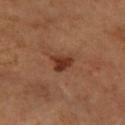Impression: No biopsy was performed on this lesion — it was imaged during a full skin examination and was not determined to be concerning. Clinical summary: A female patient aged around 65. The lesion is located on the right forearm. Automated tile analysis of the lesion measured a border-irregularity index near 4/10 and radial color variation of about 1. The software also gave an automated nevus-likeness rating near 90 out of 100 and a detector confidence of about 100 out of 100 that the crop contains a lesion. Approximately 3 mm at its widest. Cropped from a whole-body photographic skin survey; the tile spans about 15 mm.